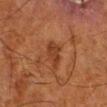biopsy status = no biopsy performed (imaged during a skin exam)
lighting = cross-polarized illumination
acquisition = ~15 mm tile from a whole-body skin photo
automated lesion analysis = border irregularity of about 3 on a 0–10 scale, a color-variation rating of about 1.5/10, and radial color variation of about 0.5
patient = male, aged 78–82
anatomic site = the right lower leg
size = about 3.5 mm